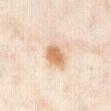notes — imaged on a skin check; not biopsied
illumination — cross-polarized illumination
TBP lesion metrics — a lesion area of about 8 mm² and an eccentricity of roughly 0.25; a nevus-likeness score of about 100/100 and a lesion-detection confidence of about 100/100
image — total-body-photography crop, ~15 mm field of view
subject — female, approximately 35 years of age
anatomic site — the abdomen
lesion size — about 3.5 mm